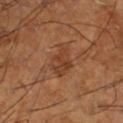Clinical impression: Captured during whole-body skin photography for melanoma surveillance; the lesion was not biopsied. Context: From the left lower leg. A male subject, aged 63–67. A close-up tile cropped from a whole-body skin photograph, about 15 mm across.